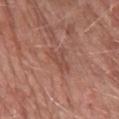Q: Is there a histopathology result?
A: catalogued during a skin exam; not biopsied
Q: What are the patient's age and sex?
A: female, aged approximately 60
Q: What kind of image is this?
A: ~15 mm tile from a whole-body skin photo
Q: Automated lesion metrics?
A: a classifier nevus-likeness of about 0/100 and lesion-presence confidence of about 100/100
Q: Lesion location?
A: the right forearm
Q: How was the tile lit?
A: white-light illumination
Q: What is the lesion's diameter?
A: ~3 mm (longest diameter)